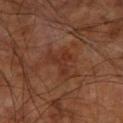A region of skin cropped from a whole-body photographic capture, roughly 15 mm wide.
The lesion is on the right upper arm.
Captured under cross-polarized illumination.
The patient is approximately 65 years of age.
The recorded lesion diameter is about 3.5 mm.